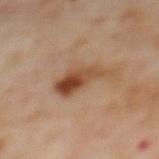Impression: Imaged during a routine full-body skin examination; the lesion was not biopsied and no histopathology is available. Context: A female patient, aged 58–62. A roughly 15 mm field-of-view crop from a total-body skin photograph. From the upper back.